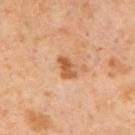image: 15 mm crop, total-body photography | patient: male, approximately 65 years of age | size: ≈3 mm | tile lighting: cross-polarized | image-analysis metrics: an area of roughly 4.5 mm², a shape eccentricity near 0.75, and a shape-asymmetry score of about 0.25 (0 = symmetric).